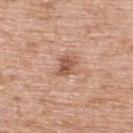Part of a total-body skin-imaging series; this lesion was reviewed on a skin check and was not flagged for biopsy. About 2.5 mm across. Imaged with white-light lighting. The subject is a male roughly 60 years of age. The total-body-photography lesion software estimated a lesion color around L≈54 a*≈22 b*≈29 in CIELAB and about 12 CIELAB-L* units darker than the surrounding skin. It also reported a border-irregularity rating of about 3/10, internal color variation of about 4 on a 0–10 scale, and a peripheral color-asymmetry measure near 1.5. A roughly 15 mm field-of-view crop from a total-body skin photograph. The lesion is on the upper back.